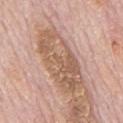notes: no biopsy performed (imaged during a skin exam) | acquisition: ~15 mm tile from a whole-body skin photo | TBP lesion metrics: an area of roughly 23 mm², an outline eccentricity of about 0.9 (0 = round, 1 = elongated), and a shape-asymmetry score of about 0.25 (0 = symmetric); roughly 11 lightness units darker than nearby skin and a normalized lesion–skin contrast near 7.5; a within-lesion color-variation index near 6/10 and radial color variation of about 2.5; an automated nevus-likeness rating near 0 out of 100 and lesion-presence confidence of about 100/100 | lighting: white-light | site: the mid back | subject: male, in their mid-70s.